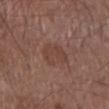biopsy status: total-body-photography surveillance lesion; no biopsy | illumination: white-light | acquisition: ~15 mm crop, total-body skin-cancer survey | body site: the front of the torso | patient: male, in their mid-50s.